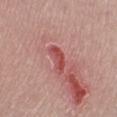The lesion was photographed on a routine skin check and not biopsied; there is no pathology result. A 15 mm close-up tile from a total-body photography series done for melanoma screening. The lesion-visualizer software estimated a footprint of about 5 mm², an eccentricity of roughly 0.9, and two-axis asymmetry of about 0.4. And it measured a nevus-likeness score of about 0/100. The tile uses white-light illumination. Approximately 3.5 mm at its widest. The patient is a male aged 58–62. The lesion is on the right lower leg.